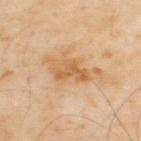Impression:
Part of a total-body skin-imaging series; this lesion was reviewed on a skin check and was not flagged for biopsy.
Background:
A lesion tile, about 15 mm wide, cut from a 3D total-body photograph. A male patient aged around 55. Measured at roughly 5.5 mm in maximum diameter. Captured under cross-polarized illumination. On the upper back. The total-body-photography lesion software estimated an area of roughly 11 mm², an eccentricity of roughly 0.85, and a shape-asymmetry score of about 0.45 (0 = symmetric). The software also gave internal color variation of about 4.5 on a 0–10 scale and radial color variation of about 1.5.